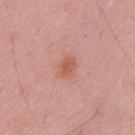follow-up = total-body-photography surveillance lesion; no biopsy
tile lighting = white-light
location = the back
automated metrics = two-axis asymmetry of about 0.25; a border-irregularity rating of about 2.5/10, a color-variation rating of about 2/10, and peripheral color asymmetry of about 0.5; a nevus-likeness score of about 80/100 and lesion-presence confidence of about 100/100
patient = male, aged around 50
diameter = ≈2.5 mm
acquisition = total-body-photography crop, ~15 mm field of view